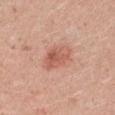workup: catalogued during a skin exam; not biopsied
lesion diameter: ≈3.5 mm
location: the arm
automated metrics: a lesion area of about 7.5 mm² and two-axis asymmetry of about 0.2; a classifier nevus-likeness of about 90/100 and a lesion-detection confidence of about 100/100
subject: male, about 25 years old
image source: total-body-photography crop, ~15 mm field of view
lighting: white-light